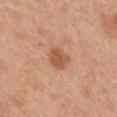Imaged during a routine full-body skin examination; the lesion was not biopsied and no histopathology is available.
Imaged with white-light lighting.
On the mid back.
Measured at roughly 3 mm in maximum diameter.
A male patient aged approximately 30.
A lesion tile, about 15 mm wide, cut from a 3D total-body photograph.